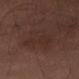Clinical impression:
Captured during whole-body skin photography for melanoma surveillance; the lesion was not biopsied.
Background:
A male subject, in their mid- to late 60s. A 15 mm close-up extracted from a 3D total-body photography capture. On the left thigh.